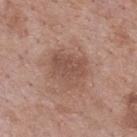biopsy status: total-body-photography surveillance lesion; no biopsy | imaging modality: total-body-photography crop, ~15 mm field of view | anatomic site: the upper back | lighting: white-light | patient: male, in their 70s.